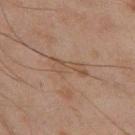Assessment: Recorded during total-body skin imaging; not selected for excision or biopsy. Context: This image is a 15 mm lesion crop taken from a total-body photograph. Captured under cross-polarized illumination. Located on the left thigh. Longest diameter approximately 4.5 mm. A male patient, aged 43–47. Automated image analysis of the tile measured lesion-presence confidence of about 60/100.